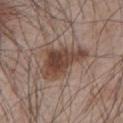Case summary:
- imaging modality: total-body-photography crop, ~15 mm field of view
- illumination: white-light illumination
- patient: male, about 65 years old
- size: about 6.5 mm
- location: the chest